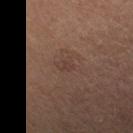diameter: about 1.5 mm | subject: female, aged 28–32 | site: the head or neck | imaging modality: total-body-photography crop, ~15 mm field of view | automated metrics: a lesion color around L≈35 a*≈16 b*≈21 in CIELAB, a lesion–skin lightness drop of about 5, and a normalized lesion–skin contrast near 5; an automated nevus-likeness rating near 0 out of 100 | illumination: cross-polarized illumination.